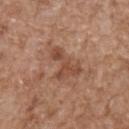<case>
  <biopsy_status>not biopsied; imaged during a skin examination</biopsy_status>
  <lesion_size>
    <long_diameter_mm_approx>5.0</long_diameter_mm_approx>
  </lesion_size>
  <site>upper back</site>
  <image>
    <source>total-body photography crop</source>
    <field_of_view_mm>15</field_of_view_mm>
  </image>
  <patient>
    <sex>male</sex>
    <age_approx>65</age_approx>
  </patient>
  <automated_metrics>
    <cielab_L>47</cielab_L>
    <cielab_a>22</cielab_a>
    <cielab_b>30</cielab_b>
    <vs_skin_darker_L>9.0</vs_skin_darker_L>
    <vs_skin_contrast_norm>7.0</vs_skin_contrast_norm>
    <border_irregularity_0_10>8.0</border_irregularity_0_10>
    <color_variation_0_10>3.0</color_variation_0_10>
  </automated_metrics>
</case>